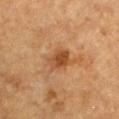Captured during whole-body skin photography for melanoma surveillance; the lesion was not biopsied.
A region of skin cropped from a whole-body photographic capture, roughly 15 mm wide.
The lesion is located on the chest.
The patient is a male approximately 85 years of age or older.
An algorithmic analysis of the crop reported a lesion area of about 6.5 mm², a shape eccentricity near 0.65, and two-axis asymmetry of about 0.25. The analysis additionally found a mean CIELAB color near L≈42 a*≈21 b*≈34, about 9 CIELAB-L* units darker than the surrounding skin, and a lesion-to-skin contrast of about 7.5 (normalized; higher = more distinct). And it measured a border-irregularity index near 2.5/10, internal color variation of about 3.5 on a 0–10 scale, and a peripheral color-asymmetry measure near 1.